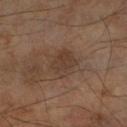Notes:
– follow-up: total-body-photography surveillance lesion; no biopsy
– body site: the arm
– TBP lesion metrics: a lesion area of about 8.5 mm² and a shape-asymmetry score of about 0.2 (0 = symmetric); an average lesion color of about L≈37 a*≈15 b*≈25 (CIELAB) and a normalized border contrast of about 6; a border-irregularity rating of about 2/10, a within-lesion color-variation index near 3/10, and peripheral color asymmetry of about 1; a nevus-likeness score of about 0/100 and a lesion-detection confidence of about 100/100
– imaging modality: total-body-photography crop, ~15 mm field of view
– patient: male, about 70 years old